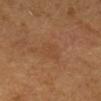On the head or neck. A female subject, about 65 years old. A roughly 15 mm field-of-view crop from a total-body skin photograph.